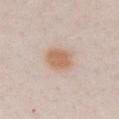follow-up: imaged on a skin check; not biopsied
site: the chest
subject: female, aged 18–22
size: ~3.5 mm (longest diameter)
automated metrics: a mean CIELAB color near L≈63 a*≈18 b*≈32, a lesion–skin lightness drop of about 10, and a lesion-to-skin contrast of about 9 (normalized; higher = more distinct)
image source: ~15 mm crop, total-body skin-cancer survey